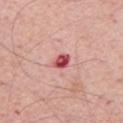Recorded during total-body skin imaging; not selected for excision or biopsy. A 15 mm close-up extracted from a 3D total-body photography capture. Imaged with white-light lighting. Automated image analysis of the tile measured a footprint of about 3.5 mm², an outline eccentricity of about 0.75 (0 = round, 1 = elongated), and two-axis asymmetry of about 0.25. A male subject aged approximately 65. The lesion's longest dimension is about 2.5 mm. On the chest.